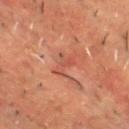workup — total-body-photography surveillance lesion; no biopsy | anatomic site — the chest | diameter — ≈3.5 mm | acquisition — ~15 mm crop, total-body skin-cancer survey | subject — male, about 50 years old | tile lighting — cross-polarized illumination | automated lesion analysis — a footprint of about 4 mm², an outline eccentricity of about 0.95 (0 = round, 1 = elongated), and two-axis asymmetry of about 0.35; about 6 CIELAB-L* units darker than the surrounding skin and a normalized border contrast of about 5.